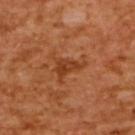Case summary:
- workup: no biopsy performed (imaged during a skin exam)
- anatomic site: the back
- image: ~15 mm crop, total-body skin-cancer survey
- lesion diameter: about 4 mm
- TBP lesion metrics: a lesion area of about 6.5 mm², a shape eccentricity near 0.75, and two-axis asymmetry of about 0.55; a border-irregularity index near 6.5/10, internal color variation of about 3.5 on a 0–10 scale, and a peripheral color-asymmetry measure near 1.5
- patient: female, in their mid- to late 50s